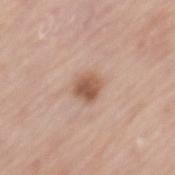Captured during whole-body skin photography for melanoma surveillance; the lesion was not biopsied. Captured under white-light illumination. From the mid back. The subject is a female in their mid- to late 60s. A region of skin cropped from a whole-body photographic capture, roughly 15 mm wide. The recorded lesion diameter is about 2.5 mm.